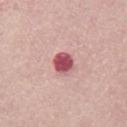The lesion was photographed on a routine skin check and not biopsied; there is no pathology result.
A male patient, about 65 years old.
A roughly 15 mm field-of-view crop from a total-body skin photograph.
The lesion is located on the front of the torso.
Automated image analysis of the tile measured a lesion area of about 5.5 mm² and a shape-asymmetry score of about 0.2 (0 = symmetric). The analysis additionally found a lesion–skin lightness drop of about 18 and a normalized border contrast of about 11.5. The analysis additionally found a border-irregularity rating of about 1.5/10, internal color variation of about 4 on a 0–10 scale, and peripheral color asymmetry of about 1.5.